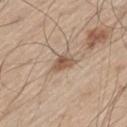Case summary:
* follow-up — imaged on a skin check; not biopsied
* patient — male, aged around 60
* anatomic site — the left thigh
* lighting — white-light illumination
* imaging modality — ~15 mm tile from a whole-body skin photo